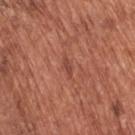Findings:
• follow-up — catalogued during a skin exam; not biopsied
• size — ~2.5 mm (longest diameter)
• illumination — white-light
• body site — the back
• patient — male, aged around 65
• TBP lesion metrics — a lesion area of about 2 mm², an outline eccentricity of about 0.95 (0 = round, 1 = elongated), and a shape-asymmetry score of about 0.3 (0 = symmetric); an average lesion color of about L≈46 a*≈28 b*≈29 (CIELAB) and a lesion–skin lightness drop of about 8; a border-irregularity index near 4/10, a within-lesion color-variation index near 0/10, and a peripheral color-asymmetry measure near 0; a classifier nevus-likeness of about 0/100
• acquisition — ~15 mm crop, total-body skin-cancer survey